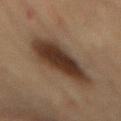Notes:
– follow-up: total-body-photography surveillance lesion; no biopsy
– body site: the mid back
– subject: female, aged 78–82
– acquisition: 15 mm crop, total-body photography
– size: ≈9.5 mm